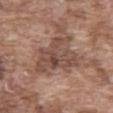Impression: Captured during whole-body skin photography for melanoma surveillance; the lesion was not biopsied. Acquisition and patient details: The lesion-visualizer software estimated a lesion area of about 20 mm², a shape eccentricity near 0.7, and a symmetry-axis asymmetry near 0.45. The software also gave border irregularity of about 5.5 on a 0–10 scale, a color-variation rating of about 5/10, and radial color variation of about 1.5. On the abdomen. A region of skin cropped from a whole-body photographic capture, roughly 15 mm wide. The recorded lesion diameter is about 7 mm. A male patient, aged around 75. Captured under white-light illumination.